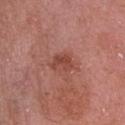biopsy_status: not biopsied; imaged during a skin examination
automated_metrics:
  area_mm2_approx: 4.5
  eccentricity: 0.75
  cielab_L: 45
  cielab_a: 27
  cielab_b: 28
  border_irregularity_0_10: 3.5
  color_variation_0_10: 2.5
  peripheral_color_asymmetry: 1.0
  nevus_likeness_0_100: 15
  lesion_detection_confidence_0_100: 100
patient:
  sex: male
  age_approx: 70
site: head or neck
image:
  source: total-body photography crop
  field_of_view_mm: 15
lesion_size:
  long_diameter_mm_approx: 3.0